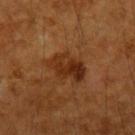Impression: The lesion was photographed on a routine skin check and not biopsied; there is no pathology result. Acquisition and patient details: Longest diameter approximately 4.5 mm. A roughly 15 mm field-of-view crop from a total-body skin photograph. Automated image analysis of the tile measured a border-irregularity index near 3.5/10, a within-lesion color-variation index near 6.5/10, and radial color variation of about 2.5. A male patient in their mid-40s. On the arm. This is a cross-polarized tile.